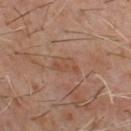Q: Was this lesion biopsied?
A: total-body-photography surveillance lesion; no biopsy
Q: What are the patient's age and sex?
A: male, in their 60s
Q: What is the anatomic site?
A: the front of the torso
Q: Automated lesion metrics?
A: a footprint of about 5 mm² and a shape-asymmetry score of about 0.45 (0 = symmetric); an automated nevus-likeness rating near 0 out of 100 and a detector confidence of about 100 out of 100 that the crop contains a lesion
Q: What is the lesion's diameter?
A: ~3.5 mm (longest diameter)
Q: How was the tile lit?
A: cross-polarized
Q: What kind of image is this?
A: total-body-photography crop, ~15 mm field of view